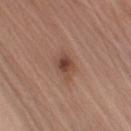– acquisition · ~15 mm tile from a whole-body skin photo
– patient · male, in their mid-70s
– body site · the arm
– automated metrics · a border-irregularity index near 2.5/10, a color-variation rating of about 5.5/10, and radial color variation of about 1.5; a detector confidence of about 100 out of 100 that the crop contains a lesion
– lesion diameter · ≈3 mm